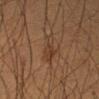The lesion-visualizer software estimated an area of roughly 5 mm² and a symmetry-axis asymmetry near 0.4. The software also gave a border-irregularity index near 5/10, a within-lesion color-variation index near 2.5/10, and radial color variation of about 1. Cropped from a total-body skin-imaging series; the visible field is about 15 mm. Captured under cross-polarized illumination. A male patient, approximately 40 years of age. About 4.5 mm across. Located on the right forearm.